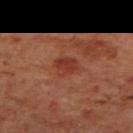Notes:
• biopsy status: no biopsy performed (imaged during a skin exam)
• lighting: cross-polarized illumination
• image source: ~15 mm tile from a whole-body skin photo
• subject: male, aged 68 to 72
• lesion size: ≈2.5 mm
• site: the mid back
• automated lesion analysis: a footprint of about 5.5 mm² and a shape-asymmetry score of about 0.25 (0 = symmetric); a mean CIELAB color near L≈36 a*≈28 b*≈29 and about 8 CIELAB-L* units darker than the surrounding skin; an automated nevus-likeness rating near 40 out of 100 and a detector confidence of about 100 out of 100 that the crop contains a lesion